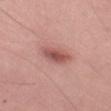| field | value |
|---|---|
| follow-up | catalogued during a skin exam; not biopsied |